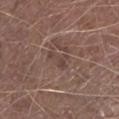workup=catalogued during a skin exam; not biopsied
tile lighting=white-light illumination
size=~3 mm (longest diameter)
subject=male, about 75 years old
location=the left lower leg
imaging modality=total-body-photography crop, ~15 mm field of view